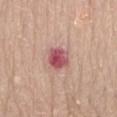Findings:
- illumination — white-light
- site — the abdomen
- subject — female, about 65 years old
- image source — ~15 mm crop, total-body skin-cancer survey